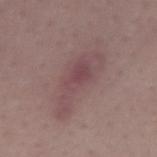No biopsy was performed on this lesion — it was imaged during a full skin examination and was not determined to be concerning.
A 15 mm close-up tile from a total-body photography series done for melanoma screening.
Measured at roughly 7.5 mm in maximum diameter.
The lesion is located on the right forearm.
The tile uses white-light illumination.
The total-body-photography lesion software estimated an automated nevus-likeness rating near 0 out of 100 and lesion-presence confidence of about 100/100.
The subject is a female in their 40s.